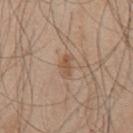No biopsy was performed on this lesion — it was imaged during a full skin examination and was not determined to be concerning.
A male subject, in their mid- to late 40s.
An algorithmic analysis of the crop reported a footprint of about 3.5 mm². It also reported border irregularity of about 3 on a 0–10 scale and a color-variation rating of about 1/10.
A roughly 15 mm field-of-view crop from a total-body skin photograph.
On the chest.
Imaged with white-light lighting.